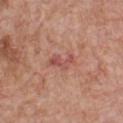follow-up = imaged on a skin check; not biopsied | anatomic site = the chest | patient = male, aged 63–67 | imaging modality = total-body-photography crop, ~15 mm field of view.